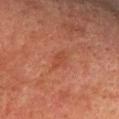Imaged during a routine full-body skin examination; the lesion was not biopsied and no histopathology is available.
Measured at roughly 2.5 mm in maximum diameter.
Cropped from a whole-body photographic skin survey; the tile spans about 15 mm.
The patient is a male aged approximately 75.
Imaged with cross-polarized lighting.
The total-body-photography lesion software estimated a lesion area of about 2.5 mm², an eccentricity of roughly 0.85, and a shape-asymmetry score of about 0.35 (0 = symmetric). The analysis additionally found a mean CIELAB color near L≈38 a*≈25 b*≈29, roughly 5 lightness units darker than nearby skin, and a normalized border contrast of about 5. It also reported border irregularity of about 3.5 on a 0–10 scale and a peripheral color-asymmetry measure near 0.
On the front of the torso.